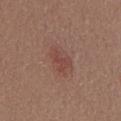workup: catalogued during a skin exam; not biopsied | subject: male, aged approximately 55 | location: the chest | image: 15 mm crop, total-body photography.